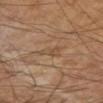Impression: Captured during whole-body skin photography for melanoma surveillance; the lesion was not biopsied. Background: Automated tile analysis of the lesion measured a nevus-likeness score of about 0/100 and lesion-presence confidence of about 95/100. A close-up tile cropped from a whole-body skin photograph, about 15 mm across. Longest diameter approximately 3 mm. The lesion is located on the right upper arm. Imaged with cross-polarized lighting.